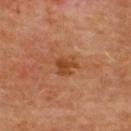Assessment: Recorded during total-body skin imaging; not selected for excision or biopsy. Context: A close-up tile cropped from a whole-body skin photograph, about 15 mm across. A female subject, aged 48 to 52. On the upper back. About 2.5 mm across.